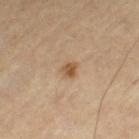workup = total-body-photography surveillance lesion; no biopsy | tile lighting = cross-polarized illumination | diameter = ~2 mm (longest diameter) | TBP lesion metrics = a border-irregularity rating of about 2/10, a color-variation rating of about 2.5/10, and radial color variation of about 1; lesion-presence confidence of about 100/100 | location = the left thigh | imaging modality = total-body-photography crop, ~15 mm field of view | subject = male, aged around 55.